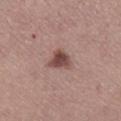Q: Is there a histopathology result?
A: catalogued during a skin exam; not biopsied
Q: What lighting was used for the tile?
A: white-light
Q: What kind of image is this?
A: 15 mm crop, total-body photography
Q: Patient demographics?
A: female, aged 63 to 67
Q: Where on the body is the lesion?
A: the leg
Q: How large is the lesion?
A: ≈3 mm